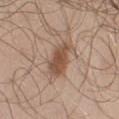Clinical impression:
Recorded during total-body skin imaging; not selected for excision or biopsy.
Image and clinical context:
Imaged with white-light lighting. Longest diameter approximately 4.5 mm. A 15 mm close-up tile from a total-body photography series done for melanoma screening. A male subject, roughly 50 years of age. From the left upper arm.